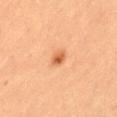Part of a total-body skin-imaging series; this lesion was reviewed on a skin check and was not flagged for biopsy.
Located on the left thigh.
A female patient, in their mid- to late 20s.
A close-up tile cropped from a whole-body skin photograph, about 15 mm across.